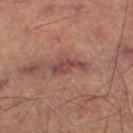Impression:
The lesion was tiled from a total-body skin photograph and was not biopsied.
Acquisition and patient details:
This is a cross-polarized tile. The lesion's longest dimension is about 4 mm. An algorithmic analysis of the crop reported a lesion area of about 4.5 mm², a shape eccentricity near 0.95, and two-axis asymmetry of about 0.4. The software also gave a mean CIELAB color near L≈44 a*≈24 b*≈22 and a lesion-to-skin contrast of about 8.5 (normalized; higher = more distinct). It also reported a classifier nevus-likeness of about 0/100 and a detector confidence of about 55 out of 100 that the crop contains a lesion. The lesion is located on the right thigh. A roughly 15 mm field-of-view crop from a total-body skin photograph. The patient is a male in their mid- to late 60s.